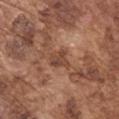Case summary:
– follow-up · total-body-photography surveillance lesion; no biopsy
– body site · the left upper arm
– imaging modality · ~15 mm crop, total-body skin-cancer survey
– lighting · white-light illumination
– automated metrics · a lesion color around L≈45 a*≈21 b*≈29 in CIELAB, about 9 CIELAB-L* units darker than the surrounding skin, and a lesion-to-skin contrast of about 6.5 (normalized; higher = more distinct); an automated nevus-likeness rating near 0 out of 100 and lesion-presence confidence of about 95/100
– patient · male, in their mid-70s